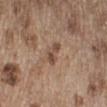Impression:
The lesion was tiled from a total-body skin photograph and was not biopsied.
Context:
A 15 mm close-up extracted from a 3D total-body photography capture. The lesion's longest dimension is about 3 mm. Located on the right lower leg. The patient is a male aged 68–72. Captured under white-light illumination. Automated tile analysis of the lesion measured border irregularity of about 4.5 on a 0–10 scale, a color-variation rating of about 1.5/10, and a peripheral color-asymmetry measure near 0. The software also gave a nevus-likeness score of about 5/100 and a detector confidence of about 100 out of 100 that the crop contains a lesion.